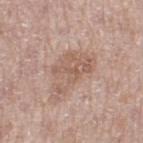Clinical impression:
This lesion was catalogued during total-body skin photography and was not selected for biopsy.
Background:
The recorded lesion diameter is about 6.5 mm. The tile uses white-light illumination. On the left thigh. A male patient, aged 63–67. A 15 mm close-up tile from a total-body photography series done for melanoma screening.